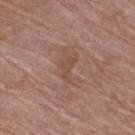{"biopsy_status": "not biopsied; imaged during a skin examination", "image": {"source": "total-body photography crop", "field_of_view_mm": 15}, "lighting": "white-light", "patient": {"sex": "female", "age_approx": 70}, "lesion_size": {"long_diameter_mm_approx": 3.0}, "automated_metrics": {"eccentricity": 0.9, "cielab_L": 48, "cielab_a": 19, "cielab_b": 27, "vs_skin_darker_L": 6.0, "vs_skin_contrast_norm": 5.0, "border_irregularity_0_10": 6.0, "color_variation_0_10": 0.0, "peripheral_color_asymmetry": 0.0, "nevus_likeness_0_100": 0, "lesion_detection_confidence_0_100": 100}, "site": "right thigh"}